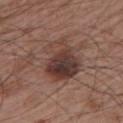{
  "biopsy_status": "not biopsied; imaged during a skin examination",
  "image": {
    "source": "total-body photography crop",
    "field_of_view_mm": 15
  },
  "site": "left upper arm",
  "lesion_size": {
    "long_diameter_mm_approx": 5.5
  },
  "automated_metrics": {
    "area_mm2_approx": 19.0,
    "eccentricity": 0.55,
    "shape_asymmetry": 0.25,
    "color_variation_0_10": 9.5,
    "peripheral_color_asymmetry": 3.0,
    "nevus_likeness_0_100": 70,
    "lesion_detection_confidence_0_100": 100
  },
  "patient": {
    "sex": "male",
    "age_approx": 65
  },
  "lighting": "white-light"
}